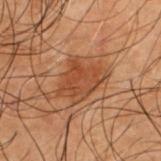<tbp_lesion>
  <biopsy_status>not biopsied; imaged during a skin examination</biopsy_status>
  <image>
    <source>total-body photography crop</source>
    <field_of_view_mm>15</field_of_view_mm>
  </image>
  <site>upper back</site>
  <lighting>cross-polarized</lighting>
  <automated_metrics>
    <cielab_L>35</cielab_L>
    <cielab_a>20</cielab_a>
    <cielab_b>29</cielab_b>
    <vs_skin_contrast_norm>7.0</vs_skin_contrast_norm>
    <nevus_likeness_0_100>85</nevus_likeness_0_100>
    <lesion_detection_confidence_0_100>100</lesion_detection_confidence_0_100>
  </automated_metrics>
  <lesion_size>
    <long_diameter_mm_approx>5.0</long_diameter_mm_approx>
  </lesion_size>
  <patient>
    <sex>male</sex>
    <age_approx>50</age_approx>
  </patient>
</tbp_lesion>